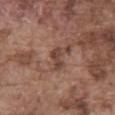Notes:
- lighting — white-light illumination
- subject — male, in their mid- to late 70s
- acquisition — total-body-photography crop, ~15 mm field of view
- lesion diameter — about 2.5 mm
- anatomic site — the front of the torso